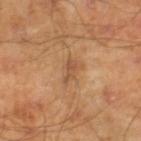biopsy status: imaged on a skin check; not biopsied
lighting: cross-polarized
image: 15 mm crop, total-body photography
size: about 3 mm
patient: male, aged 43–47
automated lesion analysis: a shape-asymmetry score of about 0.35 (0 = symmetric); a lesion color around L≈51 a*≈21 b*≈34 in CIELAB, a lesion–skin lightness drop of about 8, and a lesion-to-skin contrast of about 6 (normalized; higher = more distinct); border irregularity of about 4.5 on a 0–10 scale and a within-lesion color-variation index near 0.5/10
location: the leg